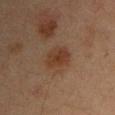The lesion was photographed on a routine skin check and not biopsied; there is no pathology result. A 15 mm crop from a total-body photograph taken for skin-cancer surveillance. A male subject, in their mid- to late 30s. The tile uses cross-polarized illumination. Automated tile analysis of the lesion measured a mean CIELAB color near L≈34 a*≈18 b*≈27 and a normalized border contrast of about 7.5. And it measured a border-irregularity rating of about 2/10 and radial color variation of about 1. The lesion is located on the chest.